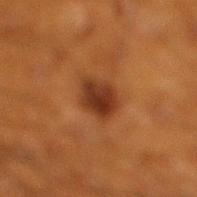Findings:
– biopsy status · imaged on a skin check; not biopsied
– patient · male, about 60 years old
– tile lighting · cross-polarized
– lesion diameter · ~3.5 mm (longest diameter)
– acquisition · ~15 mm tile from a whole-body skin photo
– site · the leg
– automated lesion analysis · a footprint of about 7.5 mm², a shape eccentricity near 0.55, and a symmetry-axis asymmetry near 0.2; a border-irregularity rating of about 2/10, a color-variation rating of about 3.5/10, and peripheral color asymmetry of about 1; an automated nevus-likeness rating near 95 out of 100 and a lesion-detection confidence of about 100/100